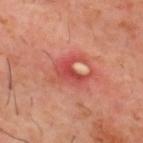Clinical impression: Imaged during a routine full-body skin examination; the lesion was not biopsied and no histopathology is available.